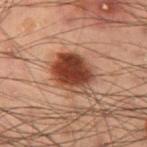Recorded during total-body skin imaging; not selected for excision or biopsy.
The lesion is located on the left thigh.
Cropped from a total-body skin-imaging series; the visible field is about 15 mm.
This is a cross-polarized tile.
A male subject, aged 53–57.